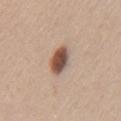The lesion was tiled from a total-body skin photograph and was not biopsied.
The patient is a female roughly 40 years of age.
On the left upper arm.
A 15 mm close-up tile from a total-body photography series done for melanoma screening.